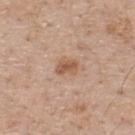biopsy status: catalogued during a skin exam; not biopsied
lighting: white-light
lesion size: about 2.5 mm
image-analysis metrics: an eccentricity of roughly 0.75 and a symmetry-axis asymmetry near 0.25; a lesion–skin lightness drop of about 10 and a normalized lesion–skin contrast near 7.5; a nevus-likeness score of about 55/100 and a detector confidence of about 100 out of 100 that the crop contains a lesion
body site: the upper back
patient: male, aged approximately 60
image: ~15 mm crop, total-body skin-cancer survey